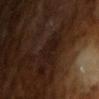No biopsy was performed on this lesion — it was imaged during a full skin examination and was not determined to be concerning.
The subject is a male aged approximately 65.
Cropped from a whole-body photographic skin survey; the tile spans about 15 mm.
This is a cross-polarized tile.
Automated image analysis of the tile measured a footprint of about 6 mm² and an eccentricity of roughly 0.3. The analysis additionally found a border-irregularity index near 4/10 and a peripheral color-asymmetry measure near 0.5.
The recorded lesion diameter is about 3 mm.